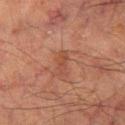subject: male, aged 63–67; image: total-body-photography crop, ~15 mm field of view; location: the right thigh.